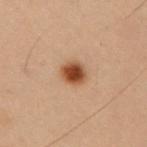Assessment: Captured during whole-body skin photography for melanoma surveillance; the lesion was not biopsied. Background: The tile uses cross-polarized illumination. A 15 mm close-up extracted from a 3D total-body photography capture. On the right upper arm. The subject is a male roughly 55 years of age.